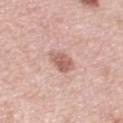Part of a total-body skin-imaging series; this lesion was reviewed on a skin check and was not flagged for biopsy. On the left upper arm. A 15 mm crop from a total-body photograph taken for skin-cancer surveillance. A female subject, aged around 50. Imaged with white-light lighting. Automated image analysis of the tile measured an area of roughly 5.5 mm² and an outline eccentricity of about 0.3 (0 = round, 1 = elongated). The software also gave a border-irregularity rating of about 2.5/10, internal color variation of about 3 on a 0–10 scale, and a peripheral color-asymmetry measure near 1.